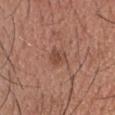Recorded during total-body skin imaging; not selected for excision or biopsy.
The patient is a male aged 53–57.
A lesion tile, about 15 mm wide, cut from a 3D total-body photograph.
The lesion is located on the head or neck.